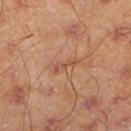Impression:
The lesion was tiled from a total-body skin photograph and was not biopsied.
Image and clinical context:
The lesion's longest dimension is about 3 mm. A male subject in their mid- to late 40s. The lesion is located on the left lower leg. This image is a 15 mm lesion crop taken from a total-body photograph.